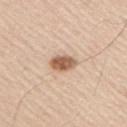Assessment:
Imaged during a routine full-body skin examination; the lesion was not biopsied and no histopathology is available.
Background:
Longest diameter approximately 3.5 mm. A male subject, aged approximately 45. Located on the upper back. This is a white-light tile. A roughly 15 mm field-of-view crop from a total-body skin photograph.